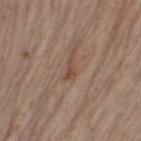Clinical summary: A 15 mm crop from a total-body photograph taken for skin-cancer surveillance. The recorded lesion diameter is about 2.5 mm. The lesion is located on the left thigh. A female subject aged 38 to 42. Captured under white-light illumination.